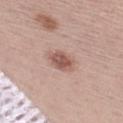Impression: The lesion was photographed on a routine skin check and not biopsied; there is no pathology result. Context: About 3.5 mm across. A male subject, aged 28 to 32. Imaged with white-light lighting. Automated image analysis of the tile measured a lesion area of about 5.5 mm² and a shape eccentricity near 0.75. It also reported a nevus-likeness score of about 85/100 and a lesion-detection confidence of about 100/100. A region of skin cropped from a whole-body photographic capture, roughly 15 mm wide.